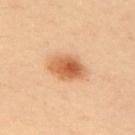Recorded during total-body skin imaging; not selected for excision or biopsy. Located on the back. Measured at roughly 4 mm in maximum diameter. The tile uses cross-polarized illumination. The total-body-photography lesion software estimated a footprint of about 9.5 mm² and an eccentricity of roughly 0.75. The analysis additionally found a mean CIELAB color near L≈61 a*≈26 b*≈40 and about 14 CIELAB-L* units darker than the surrounding skin. The software also gave a border-irregularity rating of about 1.5/10, a color-variation rating of about 6.5/10, and radial color variation of about 2. And it measured a nevus-likeness score of about 100/100 and a detector confidence of about 100 out of 100 that the crop contains a lesion. A female patient in their mid- to late 30s. A 15 mm close-up tile from a total-body photography series done for melanoma screening.